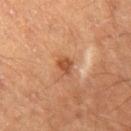Assessment: Captured during whole-body skin photography for melanoma surveillance; the lesion was not biopsied. Context: The lesion is located on the left thigh. Measured at roughly 2.5 mm in maximum diameter. The subject is a male aged around 60. This image is a 15 mm lesion crop taken from a total-body photograph.